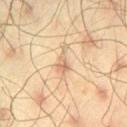workup — imaged on a skin check; not biopsied
automated lesion analysis — border irregularity of about 6 on a 0–10 scale, internal color variation of about 2.5 on a 0–10 scale, and a peripheral color-asymmetry measure near 1; a classifier nevus-likeness of about 0/100 and a lesion-detection confidence of about 80/100
size — ≈4 mm
image — ~15 mm crop, total-body skin-cancer survey
lighting — cross-polarized
location — the left thigh
subject — male, roughly 45 years of age